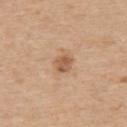Assessment:
Captured during whole-body skin photography for melanoma surveillance; the lesion was not biopsied.